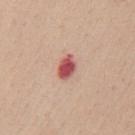Recorded during total-body skin imaging; not selected for excision or biopsy. Automated image analysis of the tile measured a border-irregularity index near 2/10, a color-variation rating of about 3/10, and peripheral color asymmetry of about 1. The software also gave an automated nevus-likeness rating near 0 out of 100 and a detector confidence of about 100 out of 100 that the crop contains a lesion. The lesion is on the back. A lesion tile, about 15 mm wide, cut from a 3D total-body photograph. This is a white-light tile. The subject is a female about 40 years old.